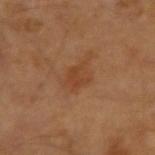automated_metrics:
  area_mm2_approx: 5.0
  eccentricity: 0.6
  shape_asymmetry: 0.25
  border_irregularity_0_10: 3.0
  color_variation_0_10: 2.5
  peripheral_color_asymmetry: 1.0
site: left arm
patient:
  sex: male
  age_approx: 65
image:
  source: total-body photography crop
  field_of_view_mm: 15